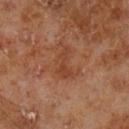The lesion was tiled from a total-body skin photograph and was not biopsied. Automated image analysis of the tile measured a mean CIELAB color near L≈41 a*≈24 b*≈32, a lesion–skin lightness drop of about 6, and a normalized border contrast of about 5.5. It also reported radial color variation of about 0.5. The analysis additionally found a classifier nevus-likeness of about 0/100. Located on the left lower leg. Cropped from a total-body skin-imaging series; the visible field is about 15 mm. A male patient, aged approximately 70. Captured under cross-polarized illumination. Approximately 4 mm at its widest.